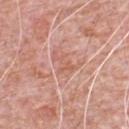biopsy_status: not biopsied; imaged during a skin examination
lesion_size:
  long_diameter_mm_approx: 3.0
site: chest
automated_metrics:
  eccentricity: 0.8
  cielab_L: 60
  cielab_a: 25
  cielab_b: 29
  vs_skin_darker_L: 6.0
  border_irregularity_0_10: 9.0
  color_variation_0_10: 0.0
lighting: white-light
patient:
  sex: male
  age_approx: 80
image:
  source: total-body photography crop
  field_of_view_mm: 15